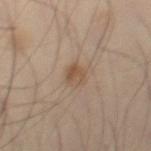Q: Was a biopsy performed?
A: catalogued during a skin exam; not biopsied
Q: Who is the patient?
A: male, roughly 50 years of age
Q: Where on the body is the lesion?
A: the lower back
Q: What kind of image is this?
A: total-body-photography crop, ~15 mm field of view